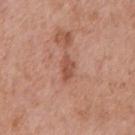biopsy status: total-body-photography surveillance lesion; no biopsy | body site: the chest | size: about 3 mm | patient: male, approximately 70 years of age | acquisition: total-body-photography crop, ~15 mm field of view.